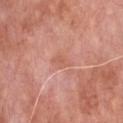The lesion was photographed on a routine skin check and not biopsied; there is no pathology result. Located on the chest. A 15 mm close-up tile from a total-body photography series done for melanoma screening. The subject is a male about 80 years old.